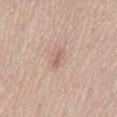  biopsy_status: not biopsied; imaged during a skin examination
  patient:
    sex: male
    age_approx: 45
  site: lower back
  image:
    source: total-body photography crop
    field_of_view_mm: 15